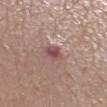Assessment:
Recorded during total-body skin imaging; not selected for excision or biopsy.
Acquisition and patient details:
The recorded lesion diameter is about 2.5 mm. A female subject, aged 48–52. A 15 mm close-up extracted from a 3D total-body photography capture. From the right lower leg. The tile uses white-light illumination.